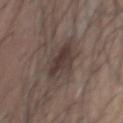Imaged during a routine full-body skin examination; the lesion was not biopsied and no histopathology is available. Cropped from a whole-body photographic skin survey; the tile spans about 15 mm. On the chest. The tile uses white-light illumination. The patient is a male in their mid-50s. About 5.5 mm across.